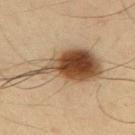<tbp_lesion>
<biopsy_status>not biopsied; imaged during a skin examination</biopsy_status>
<image>
  <source>total-body photography crop</source>
  <field_of_view_mm>15</field_of_view_mm>
</image>
<lesion_size>
  <long_diameter_mm_approx>11.5</long_diameter_mm_approx>
</lesion_size>
<site>right upper arm</site>
<lighting>cross-polarized</lighting>
<patient>
  <sex>male</sex>
  <age_approx>35</age_approx>
</patient>
</tbp_lesion>